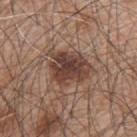Part of a total-body skin-imaging series; this lesion was reviewed on a skin check and was not flagged for biopsy. Cropped from a total-body skin-imaging series; the visible field is about 15 mm. Imaged with white-light lighting. A male patient, aged 48–52. The lesion is located on the upper back. Approximately 5.5 mm at its widest.